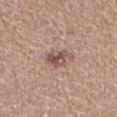Imaged during a routine full-body skin examination; the lesion was not biopsied and no histopathology is available. Cropped from a total-body skin-imaging series; the visible field is about 15 mm. This is a white-light tile. Longest diameter approximately 3 mm. The subject is a female approximately 40 years of age. The lesion is located on the leg.